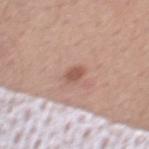This lesion was catalogued during total-body skin photography and was not selected for biopsy.
Located on the chest.
A close-up tile cropped from a whole-body skin photograph, about 15 mm across.
A male subject roughly 55 years of age.
Longest diameter approximately 2.5 mm.